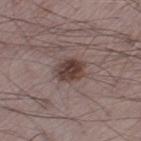Captured during whole-body skin photography for melanoma surveillance; the lesion was not biopsied. A 15 mm close-up tile from a total-body photography series done for melanoma screening. Located on the left thigh. A male patient approximately 70 years of age. Measured at roughly 3.5 mm in maximum diameter.